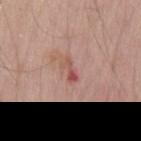{
  "site": "back",
  "lesion_size": {
    "long_diameter_mm_approx": 3.0
  },
  "lighting": "white-light",
  "image": {
    "source": "total-body photography crop",
    "field_of_view_mm": 15
  },
  "automated_metrics": {
    "border_irregularity_0_10": 4.5,
    "color_variation_0_10": 0.5,
    "peripheral_color_asymmetry": 0.0,
    "nevus_likeness_0_100": 0,
    "lesion_detection_confidence_0_100": 100
  },
  "patient": {
    "sex": "male",
    "age_approx": 70
  }
}